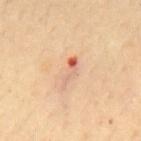Q: Is there a histopathology result?
A: no biopsy performed (imaged during a skin exam)
Q: What lighting was used for the tile?
A: cross-polarized
Q: Automated lesion metrics?
A: a footprint of about 4 mm², a shape eccentricity near 0.95, and two-axis asymmetry of about 0.45
Q: Where on the body is the lesion?
A: the mid back
Q: What is the imaging modality?
A: ~15 mm tile from a whole-body skin photo
Q: Patient demographics?
A: male, roughly 30 years of age